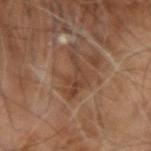Findings:
- acquisition: total-body-photography crop, ~15 mm field of view
- image-analysis metrics: internal color variation of about 3.5 on a 0–10 scale and peripheral color asymmetry of about 1.5; a classifier nevus-likeness of about 0/100 and a detector confidence of about 70 out of 100 that the crop contains a lesion
- patient: male, aged 58 to 62
- lesion diameter: ≈5.5 mm
- anatomic site: the right arm
- lighting: cross-polarized illumination